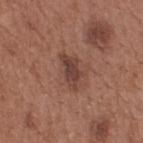Clinical impression: Recorded during total-body skin imaging; not selected for excision or biopsy. Acquisition and patient details: On the upper back. Measured at roughly 4 mm in maximum diameter. Captured under white-light illumination. A 15 mm crop from a total-body photograph taken for skin-cancer surveillance. A male subject, aged around 65. Automated image analysis of the tile measured a mean CIELAB color near L≈41 a*≈21 b*≈24, a lesion–skin lightness drop of about 10, and a lesion-to-skin contrast of about 8 (normalized; higher = more distinct).